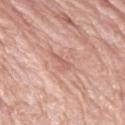Captured during whole-body skin photography for melanoma surveillance; the lesion was not biopsied.
From the left upper arm.
The lesion's longest dimension is about 2.5 mm.
The patient is a male roughly 80 years of age.
A close-up tile cropped from a whole-body skin photograph, about 15 mm across.
Imaged with white-light lighting.